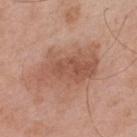<lesion>
<biopsy_status>not biopsied; imaged during a skin examination</biopsy_status>
<image>
  <source>total-body photography crop</source>
  <field_of_view_mm>15</field_of_view_mm>
</image>
<automated_metrics>
  <cielab_L>54</cielab_L>
  <cielab_a>22</cielab_a>
  <cielab_b>29</cielab_b>
  <vs_skin_darker_L>9.0</vs_skin_darker_L>
  <vs_skin_contrast_norm>6.5</vs_skin_contrast_norm>
  <nevus_likeness_0_100>20</nevus_likeness_0_100>
  <lesion_detection_confidence_0_100>100</lesion_detection_confidence_0_100>
</automated_metrics>
<lighting>white-light</lighting>
<patient>
  <sex>male</sex>
  <age_approx>55</age_approx>
</patient>
<lesion_size>
  <long_diameter_mm_approx>7.5</long_diameter_mm_approx>
</lesion_size>
<site>upper back</site>
</lesion>